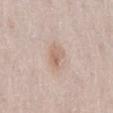The lesion was photographed on a routine skin check and not biopsied; there is no pathology result. The lesion is on the back. A female subject aged approximately 40. Approximately 2.5 mm at its widest. An algorithmic analysis of the crop reported an area of roughly 5 mm², a shape eccentricity near 0.7, and two-axis asymmetry of about 0.4. The analysis additionally found a classifier nevus-likeness of about 35/100 and a lesion-detection confidence of about 100/100. A 15 mm crop from a total-body photograph taken for skin-cancer surveillance. This is a white-light tile.